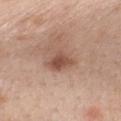<case>
<biopsy_status>not biopsied; imaged during a skin examination</biopsy_status>
</case>